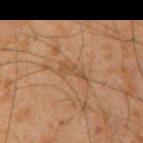{"biopsy_status": "not biopsied; imaged during a skin examination", "patient": {"sex": "male", "age_approx": 35}, "lighting": "cross-polarized", "image": {"source": "total-body photography crop", "field_of_view_mm": 15}, "site": "right upper arm"}